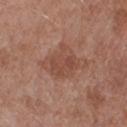Part of a total-body skin-imaging series; this lesion was reviewed on a skin check and was not flagged for biopsy. The lesion-visualizer software estimated a lesion color around L≈48 a*≈22 b*≈28 in CIELAB, roughly 7 lightness units darker than nearby skin, and a normalized lesion–skin contrast near 5.5. It also reported a border-irregularity index near 3/10, a color-variation rating of about 3/10, and peripheral color asymmetry of about 1. It also reported a nevus-likeness score of about 0/100 and a detector confidence of about 100 out of 100 that the crop contains a lesion. The tile uses white-light illumination. From the right forearm. A lesion tile, about 15 mm wide, cut from a 3D total-body photograph. A female subject, aged 48–52.